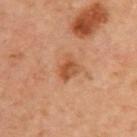Clinical impression: Part of a total-body skin-imaging series; this lesion was reviewed on a skin check and was not flagged for biopsy. Context: The patient is a male aged 63 to 67. Cropped from a whole-body photographic skin survey; the tile spans about 15 mm. The lesion is located on the upper back. The tile uses cross-polarized illumination. The lesion's longest dimension is about 3 mm. Automated image analysis of the tile measured an area of roughly 5 mm² and a shape-asymmetry score of about 0.3 (0 = symmetric). It also reported a mean CIELAB color near L≈53 a*≈26 b*≈38, roughly 10 lightness units darker than nearby skin, and a lesion-to-skin contrast of about 7.5 (normalized; higher = more distinct). And it measured a nevus-likeness score of about 70/100 and lesion-presence confidence of about 100/100.